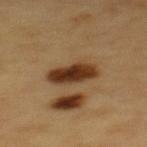follow-up: imaged on a skin check; not biopsied | subject: male, aged approximately 60 | diameter: about 5 mm | image: ~15 mm crop, total-body skin-cancer survey | anatomic site: the mid back | lighting: cross-polarized.